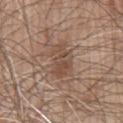No biopsy was performed on this lesion — it was imaged during a full skin examination and was not determined to be concerning.
From the chest.
The patient is a male aged 78–82.
The total-body-photography lesion software estimated a lesion area of about 8 mm², an outline eccentricity of about 0.8 (0 = round, 1 = elongated), and a shape-asymmetry score of about 0.3 (0 = symmetric). The software also gave peripheral color asymmetry of about 1.5. It also reported an automated nevus-likeness rating near 5 out of 100 and a lesion-detection confidence of about 100/100.
Imaged with white-light lighting.
A 15 mm crop from a total-body photograph taken for skin-cancer surveillance.